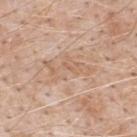  biopsy_status: not biopsied; imaged during a skin examination
  image:
    source: total-body photography crop
    field_of_view_mm: 15
  automated_metrics:
    lesion_detection_confidence_0_100: 70
  patient:
    sex: male
    age_approx: 50
  site: upper back